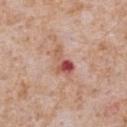Part of a total-body skin-imaging series; this lesion was reviewed on a skin check and was not flagged for biopsy.
A male subject approximately 65 years of age.
On the chest.
A 15 mm close-up tile from a total-body photography series done for melanoma screening.